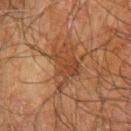{"biopsy_status": "not biopsied; imaged during a skin examination", "patient": {"sex": "male", "age_approx": 70}, "lighting": "cross-polarized", "image": {"source": "total-body photography crop", "field_of_view_mm": 15}, "site": "right forearm"}